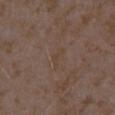Assessment: No biopsy was performed on this lesion — it was imaged during a full skin examination and was not determined to be concerning. Context: The lesion is on the right forearm. Imaged with white-light lighting. A lesion tile, about 15 mm wide, cut from a 3D total-body photograph. The patient is a female approximately 35 years of age.